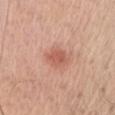biopsy status: imaged on a skin check; not biopsied
site: the front of the torso
patient: male, aged around 60
size: about 3 mm
automated metrics: an average lesion color of about L≈58 a*≈26 b*≈30 (CIELAB), a lesion–skin lightness drop of about 9, and a lesion-to-skin contrast of about 6.5 (normalized; higher = more distinct); a classifier nevus-likeness of about 95/100 and a lesion-detection confidence of about 100/100
tile lighting: white-light illumination
imaging modality: total-body-photography crop, ~15 mm field of view